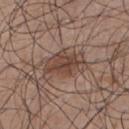Impression: Part of a total-body skin-imaging series; this lesion was reviewed on a skin check and was not flagged for biopsy. Clinical summary: Measured at roughly 5.5 mm in maximum diameter. A 15 mm close-up tile from a total-body photography series done for melanoma screening. On the back. The total-body-photography lesion software estimated a lesion area of about 14 mm², a shape eccentricity near 0.8, and a shape-asymmetry score of about 0.25 (0 = symmetric). The software also gave a mean CIELAB color near L≈44 a*≈17 b*≈25, a lesion–skin lightness drop of about 9, and a normalized border contrast of about 7.5. It also reported border irregularity of about 3.5 on a 0–10 scale, a color-variation rating of about 4.5/10, and peripheral color asymmetry of about 1.5. And it measured a nevus-likeness score of about 65/100 and a detector confidence of about 100 out of 100 that the crop contains a lesion. A male patient aged approximately 50.